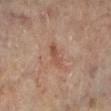This lesion was catalogued during total-body skin photography and was not selected for biopsy. The recorded lesion diameter is about 3.5 mm. The lesion is located on the leg. Cropped from a total-body skin-imaging series; the visible field is about 15 mm. A female patient aged around 60. The tile uses cross-polarized illumination.